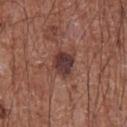Located on the chest.
The patient is a male in their mid-70s.
A 15 mm close-up tile from a total-body photography series done for melanoma screening.
This is a white-light tile.